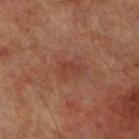Assessment:
No biopsy was performed on this lesion — it was imaged during a full skin examination and was not determined to be concerning.
Background:
Captured under cross-polarized illumination. On the right lower leg. A male subject about 70 years old. A region of skin cropped from a whole-body photographic capture, roughly 15 mm wide.